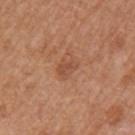Q: Was this lesion biopsied?
A: total-body-photography surveillance lesion; no biopsy
Q: What kind of image is this?
A: total-body-photography crop, ~15 mm field of view
Q: What did automated image analysis measure?
A: an area of roughly 4.5 mm², a shape eccentricity near 0.75, and a symmetry-axis asymmetry near 0.4; border irregularity of about 4 on a 0–10 scale and a within-lesion color-variation index near 1.5/10; an automated nevus-likeness rating near 5 out of 100 and a lesion-detection confidence of about 100/100
Q: Illumination type?
A: white-light
Q: What are the patient's age and sex?
A: male, in their mid-60s
Q: Lesion location?
A: the arm